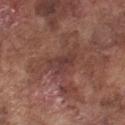  biopsy_status: not biopsied; imaged during a skin examination
  automated_metrics:
    vs_skin_contrast_norm: 6.0
    border_irregularity_0_10: 4.5
    color_variation_0_10: 2.5
    peripheral_color_asymmetry: 0.5
    lesion_detection_confidence_0_100: 80
  lesion_size:
    long_diameter_mm_approx: 4.0
  image:
    source: total-body photography crop
    field_of_view_mm: 15
  site: chest
  patient:
    sex: male
    age_approx: 75
  lighting: white-light